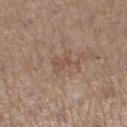Assessment: Recorded during total-body skin imaging; not selected for excision or biopsy. Background: This is a white-light tile. This image is a 15 mm lesion crop taken from a total-body photograph. A female subject, approximately 45 years of age. Automated image analysis of the tile measured a footprint of about 4 mm², a shape eccentricity near 0.85, and a symmetry-axis asymmetry near 0.6. And it measured a lesion color around L≈50 a*≈18 b*≈27 in CIELAB and a lesion–skin lightness drop of about 7. The analysis additionally found a nevus-likeness score of about 0/100 and a detector confidence of about 100 out of 100 that the crop contains a lesion. On the leg. Measured at roughly 3.5 mm in maximum diameter.